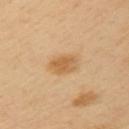The lesion was tiled from a total-body skin photograph and was not biopsied. Imaged with cross-polarized lighting. A male patient roughly 40 years of age. A roughly 15 mm field-of-view crop from a total-body skin photograph. Measured at roughly 3 mm in maximum diameter. The lesion-visualizer software estimated an area of roughly 6 mm² and two-axis asymmetry of about 0.25. The software also gave a lesion color around L≈60 a*≈19 b*≈41 in CIELAB and a normalized border contrast of about 7. On the arm.